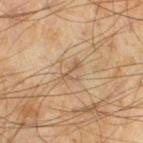Impression:
Imaged during a routine full-body skin examination; the lesion was not biopsied and no histopathology is available.
Acquisition and patient details:
A lesion tile, about 15 mm wide, cut from a 3D total-body photograph. Captured under cross-polarized illumination. Located on the left thigh. Measured at roughly 3 mm in maximum diameter. A male patient about 45 years old.